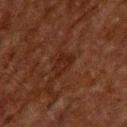biopsy_status: not biopsied; imaged during a skin examination
patient:
  sex: male
  age_approx: 60
site: upper back
automated_metrics:
  eccentricity: 0.75
  shape_asymmetry: 0.35
  cielab_L: 18
  cielab_a: 18
  cielab_b: 22
  vs_skin_darker_L: 5.0
  border_irregularity_0_10: 3.5
  color_variation_0_10: 2.0
lighting: cross-polarized
lesion_size:
  long_diameter_mm_approx: 3.0
image:
  source: total-body photography crop
  field_of_view_mm: 15